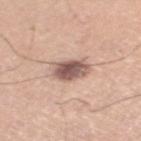Case summary:
- workup — no biopsy performed (imaged during a skin exam)
- subject — male, about 35 years old
- anatomic site — the left lower leg
- image source — ~15 mm crop, total-body skin-cancer survey